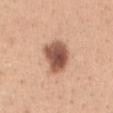workup: total-body-photography surveillance lesion; no biopsy
patient: female, in their 30s
image: total-body-photography crop, ~15 mm field of view
size: about 4 mm
body site: the abdomen
illumination: white-light illumination
TBP lesion metrics: a lesion–skin lightness drop of about 19; lesion-presence confidence of about 100/100